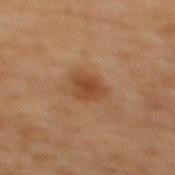biopsy status: no biopsy performed (imaged during a skin exam)
imaging modality: total-body-photography crop, ~15 mm field of view
automated lesion analysis: a lesion area of about 6 mm², an eccentricity of roughly 0.5, and two-axis asymmetry of about 0.25; a border-irregularity rating of about 2/10, a color-variation rating of about 2/10, and a peripheral color-asymmetry measure near 0.5; lesion-presence confidence of about 100/100
site: the mid back
subject: male, aged 68 to 72
size: about 3 mm